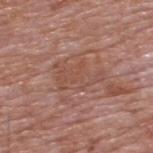notes = no biopsy performed (imaged during a skin exam) | lighting = white-light illumination | automated metrics = a symmetry-axis asymmetry near 0.45; a lesion color around L≈50 a*≈22 b*≈27 in CIELAB and a lesion–skin lightness drop of about 6; a nevus-likeness score of about 0/100 and a detector confidence of about 80 out of 100 that the crop contains a lesion | location = the upper back | subject = male, aged around 60 | imaging modality = ~15 mm tile from a whole-body skin photo.